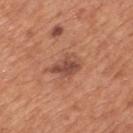body site: the mid back | subject: male, about 65 years old | image-analysis metrics: a border-irregularity rating of about 3/10, internal color variation of about 4 on a 0–10 scale, and peripheral color asymmetry of about 1.5; a classifier nevus-likeness of about 35/100 | lighting: white-light | imaging modality: 15 mm crop, total-body photography | lesion diameter: ≈3.5 mm.